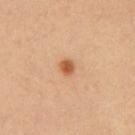Impression:
Part of a total-body skin-imaging series; this lesion was reviewed on a skin check and was not flagged for biopsy.
Context:
The lesion's longest dimension is about 2 mm. Located on the chest. This is a cross-polarized tile. Cropped from a total-body skin-imaging series; the visible field is about 15 mm. The patient is a male aged 53–57.